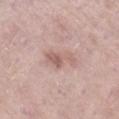Assessment: Captured during whole-body skin photography for melanoma surveillance; the lesion was not biopsied. Clinical summary: The lesion is located on the right lower leg. This is a white-light tile. A close-up tile cropped from a whole-body skin photograph, about 15 mm across. Automated tile analysis of the lesion measured a color-variation rating of about 1.5/10. And it measured a classifier nevus-likeness of about 5/100. The patient is a female aged 68 to 72.